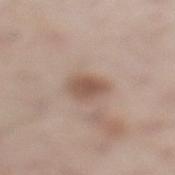| key | value |
|---|---|
| biopsy status | total-body-photography surveillance lesion; no biopsy |
| lighting | white-light illumination |
| image source | 15 mm crop, total-body photography |
| location | the left lower leg |
| diameter | about 3.5 mm |
| subject | male, aged 58–62 |
| TBP lesion metrics | a border-irregularity index near 2.5/10, a within-lesion color-variation index near 2.5/10, and a peripheral color-asymmetry measure near 0.5 |